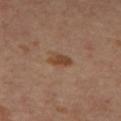Assessment:
This lesion was catalogued during total-body skin photography and was not selected for biopsy.
Acquisition and patient details:
A lesion tile, about 15 mm wide, cut from a 3D total-body photograph. Longest diameter approximately 3 mm. The total-body-photography lesion software estimated a classifier nevus-likeness of about 85/100 and lesion-presence confidence of about 100/100. On the mid back. A female patient, approximately 45 years of age.